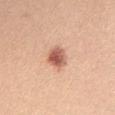A female patient approximately 45 years of age. The lesion is on the chest. The tile uses white-light illumination. An algorithmic analysis of the crop reported an automated nevus-likeness rating near 95 out of 100 and a detector confidence of about 100 out of 100 that the crop contains a lesion. This image is a 15 mm lesion crop taken from a total-body photograph.